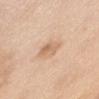Part of a total-body skin-imaging series; this lesion was reviewed on a skin check and was not flagged for biopsy.